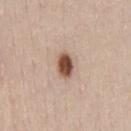Captured during whole-body skin photography for melanoma surveillance; the lesion was not biopsied. This is a white-light tile. The lesion is located on the chest. The lesion-visualizer software estimated a lesion area of about 5 mm², a shape eccentricity near 0.75, and a symmetry-axis asymmetry near 0.2. Approximately 3 mm at its widest. A close-up tile cropped from a whole-body skin photograph, about 15 mm across. A male patient about 40 years old.